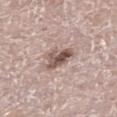Impression:
Captured during whole-body skin photography for melanoma surveillance; the lesion was not biopsied.
Context:
A male patient about 70 years old. A 15 mm close-up extracted from a 3D total-body photography capture. The lesion is on the right leg. Measured at roughly 3.5 mm in maximum diameter.